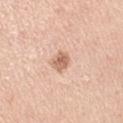No biopsy was performed on this lesion — it was imaged during a full skin examination and was not determined to be concerning. About 2.5 mm across. Automated tile analysis of the lesion measured a footprint of about 4.5 mm², a shape eccentricity near 0.65, and a symmetry-axis asymmetry near 0.2. The software also gave a lesion color around L≈64 a*≈22 b*≈31 in CIELAB, a lesion–skin lightness drop of about 13, and a lesion-to-skin contrast of about 7.5 (normalized; higher = more distinct). The software also gave a border-irregularity rating of about 2/10, internal color variation of about 4 on a 0–10 scale, and peripheral color asymmetry of about 1.5. The software also gave a classifier nevus-likeness of about 70/100 and a detector confidence of about 100 out of 100 that the crop contains a lesion. This image is a 15 mm lesion crop taken from a total-body photograph. The patient is a female aged 43–47. Imaged with white-light lighting. The lesion is on the arm.